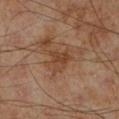No biopsy was performed on this lesion — it was imaged during a full skin examination and was not determined to be concerning. The lesion is on the left lower leg. The tile uses cross-polarized illumination. The patient is a male roughly 70 years of age. This image is a 15 mm lesion crop taken from a total-body photograph.